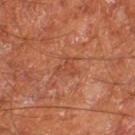Q: Is there a histopathology result?
A: total-body-photography surveillance lesion; no biopsy
Q: Lesion location?
A: the right thigh
Q: What are the patient's age and sex?
A: male, in their mid-60s
Q: How was the tile lit?
A: cross-polarized
Q: What is the imaging modality?
A: ~15 mm tile from a whole-body skin photo